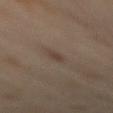Assessment:
No biopsy was performed on this lesion — it was imaged during a full skin examination and was not determined to be concerning.
Clinical summary:
A roughly 15 mm field-of-view crop from a total-body skin photograph. The patient is a male approximately 65 years of age. On the mid back.